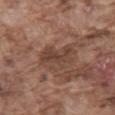Case summary:
- illumination · white-light
- patient · male, in their mid-70s
- TBP lesion metrics · an area of roughly 13 mm² and an eccentricity of roughly 0.75; a lesion color around L≈43 a*≈19 b*≈25 in CIELAB, about 9 CIELAB-L* units darker than the surrounding skin, and a lesion-to-skin contrast of about 7 (normalized; higher = more distinct); border irregularity of about 3 on a 0–10 scale, a color-variation rating of about 4.5/10, and radial color variation of about 1.5
- anatomic site · the mid back
- imaging modality · ~15 mm crop, total-body skin-cancer survey